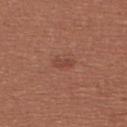Clinical impression: The lesion was tiled from a total-body skin photograph and was not biopsied. Acquisition and patient details: Located on the upper back. A roughly 15 mm field-of-view crop from a total-body skin photograph. A female subject in their mid- to late 20s.